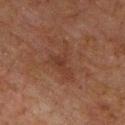follow-up: imaged on a skin check; not biopsied
lighting: cross-polarized illumination
acquisition: ~15 mm tile from a whole-body skin photo
site: the chest
subject: male, approximately 60 years of age
lesion size: ~5 mm (longest diameter)
TBP lesion metrics: a footprint of about 8.5 mm², an outline eccentricity of about 0.75 (0 = round, 1 = elongated), and a symmetry-axis asymmetry near 0.65; a nevus-likeness score of about 0/100